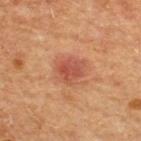Impression: The lesion was photographed on a routine skin check and not biopsied; there is no pathology result.